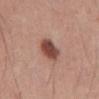• workup · total-body-photography surveillance lesion; no biopsy
• subject · male, aged approximately 55
• diameter · ≈3.5 mm
• automated metrics · a footprint of about 7.5 mm², an outline eccentricity of about 0.5 (0 = round, 1 = elongated), and two-axis asymmetry of about 0.15; a mean CIELAB color near L≈47 a*≈23 b*≈26 and a lesion–skin lightness drop of about 15; a border-irregularity rating of about 1.5/10, internal color variation of about 3.5 on a 0–10 scale, and a peripheral color-asymmetry measure near 1; a nevus-likeness score of about 100/100 and a lesion-detection confidence of about 100/100
• image · ~15 mm tile from a whole-body skin photo
• location · the abdomen
• illumination · white-light illumination